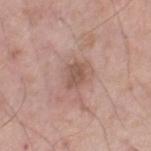The lesion was photographed on a routine skin check and not biopsied; there is no pathology result. Located on the leg. Approximately 3 mm at its widest. A 15 mm close-up extracted from a 3D total-body photography capture. The lesion-visualizer software estimated a lesion area of about 4.5 mm² and two-axis asymmetry of about 0.35. And it measured a lesion color around L≈53 a*≈18 b*≈25 in CIELAB and about 9 CIELAB-L* units darker than the surrounding skin. The software also gave a border-irregularity index near 3.5/10, a color-variation rating of about 1.5/10, and peripheral color asymmetry of about 0.5. A male subject, approximately 70 years of age. Captured under white-light illumination.